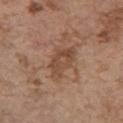Q: Was a biopsy performed?
A: total-body-photography surveillance lesion; no biopsy
Q: What lighting was used for the tile?
A: white-light
Q: What are the patient's age and sex?
A: female, aged approximately 65
Q: How was this image acquired?
A: 15 mm crop, total-body photography
Q: Automated lesion metrics?
A: a classifier nevus-likeness of about 0/100 and a lesion-detection confidence of about 100/100
Q: Where on the body is the lesion?
A: the arm
Q: What is the lesion's diameter?
A: ≈5 mm